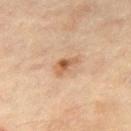Imaged during a routine full-body skin examination; the lesion was not biopsied and no histopathology is available. The tile uses cross-polarized illumination. A male patient about 65 years old. A 15 mm crop from a total-body photograph taken for skin-cancer surveillance. From the leg.